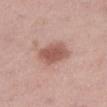Impression: The lesion was photographed on a routine skin check and not biopsied; there is no pathology result. Background: The subject is a female roughly 45 years of age. Cropped from a whole-body photographic skin survey; the tile spans about 15 mm. This is a white-light tile. Measured at roughly 4 mm in maximum diameter. An algorithmic analysis of the crop reported a symmetry-axis asymmetry near 0.15. The software also gave a nevus-likeness score of about 90/100 and a detector confidence of about 100 out of 100 that the crop contains a lesion. On the right thigh.